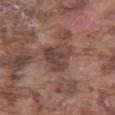| field | value |
|---|---|
| workup | no biopsy performed (imaged during a skin exam) |
| site | the left thigh |
| patient | male, aged around 75 |
| automated lesion analysis | a shape-asymmetry score of about 0.35 (0 = symmetric); a mean CIELAB color near L≈42 a*≈19 b*≈22, about 9 CIELAB-L* units darker than the surrounding skin, and a normalized border contrast of about 7.5; a border-irregularity rating of about 3.5/10, a within-lesion color-variation index near 4.5/10, and radial color variation of about 1.5; a detector confidence of about 75 out of 100 that the crop contains a lesion |
| image | total-body-photography crop, ~15 mm field of view |
| lighting | white-light |